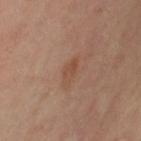| feature | finding |
|---|---|
| follow-up | no biopsy performed (imaged during a skin exam) |
| anatomic site | the left upper arm |
| diameter | ~3 mm (longest diameter) |
| patient | female, aged around 65 |
| lighting | cross-polarized |
| automated metrics | an area of roughly 4 mm² and a shape-asymmetry score of about 0.2 (0 = symmetric); a border-irregularity index near 2/10, internal color variation of about 3 on a 0–10 scale, and a peripheral color-asymmetry measure near 1 |
| image source | total-body-photography crop, ~15 mm field of view |